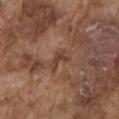{
  "image": {
    "source": "total-body photography crop",
    "field_of_view_mm": 15
  },
  "patient": {
    "sex": "male",
    "age_approx": 75
  },
  "site": "left upper arm",
  "automated_metrics": {
    "cielab_L": 41,
    "cielab_a": 19,
    "cielab_b": 27,
    "vs_skin_darker_L": 8.0,
    "vs_skin_contrast_norm": 6.5,
    "nevus_likeness_0_100": 0,
    "lesion_detection_confidence_0_100": 70
  },
  "lighting": "white-light",
  "lesion_size": {
    "long_diameter_mm_approx": 3.0
  }
}